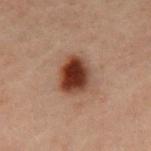Clinical summary:
From the abdomen. The total-body-photography lesion software estimated a footprint of about 11 mm², a shape eccentricity near 0.7, and a shape-asymmetry score of about 0.15 (0 = symmetric). The analysis additionally found a lesion color around L≈28 a*≈19 b*≈23 in CIELAB, a lesion–skin lightness drop of about 15, and a normalized lesion–skin contrast near 14. The analysis additionally found a border-irregularity index near 1.5/10 and peripheral color asymmetry of about 1.5. The software also gave a nevus-likeness score of about 100/100. The lesion's longest dimension is about 4.5 mm. This image is a 15 mm lesion crop taken from a total-body photograph. The tile uses cross-polarized illumination. A male patient roughly 60 years of age.